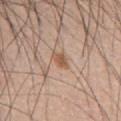follow-up = no biopsy performed (imaged during a skin exam)
location = the abdomen
lesion size = ~2.5 mm (longest diameter)
subject = male, about 65 years old
image source = total-body-photography crop, ~15 mm field of view
tile lighting = white-light